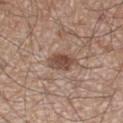Recorded during total-body skin imaging; not selected for excision or biopsy. The total-body-photography lesion software estimated a footprint of about 6.5 mm² and a symmetry-axis asymmetry near 0.2. The analysis additionally found an average lesion color of about L≈47 a*≈19 b*≈26 (CIELAB) and a normalized lesion–skin contrast near 8.5. And it measured a border-irregularity rating of about 2/10, a color-variation rating of about 3.5/10, and radial color variation of about 1. The software also gave a classifier nevus-likeness of about 90/100. Measured at roughly 3.5 mm in maximum diameter. This is a white-light tile. On the right thigh. A close-up tile cropped from a whole-body skin photograph, about 15 mm across. A male subject, approximately 60 years of age.